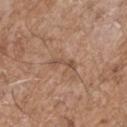Impression:
Part of a total-body skin-imaging series; this lesion was reviewed on a skin check and was not flagged for biopsy.
Acquisition and patient details:
The lesion is on the left upper arm. A roughly 15 mm field-of-view crop from a total-body skin photograph. The recorded lesion diameter is about 3.5 mm. This is a white-light tile. The subject is a male aged around 70. The total-body-photography lesion software estimated a mean CIELAB color near L≈51 a*≈18 b*≈29 and roughly 8 lightness units darker than nearby skin. And it measured a border-irregularity rating of about 5/10, internal color variation of about 0 on a 0–10 scale, and a peripheral color-asymmetry measure near 0. The software also gave an automated nevus-likeness rating near 0 out of 100.